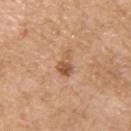Q: Was a biopsy performed?
A: catalogued during a skin exam; not biopsied
Q: Who is the patient?
A: male, aged 58 to 62
Q: Where on the body is the lesion?
A: the right upper arm
Q: What is the imaging modality?
A: ~15 mm crop, total-body skin-cancer survey